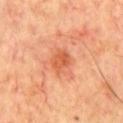workup: imaged on a skin check; not biopsied
body site: the chest
automated lesion analysis: a mean CIELAB color near L≈57 a*≈31 b*≈40, a lesion–skin lightness drop of about 9, and a lesion-to-skin contrast of about 6.5 (normalized; higher = more distinct); a nevus-likeness score of about 70/100 and lesion-presence confidence of about 100/100
illumination: cross-polarized illumination
acquisition: total-body-photography crop, ~15 mm field of view
patient: male, aged 68 to 72